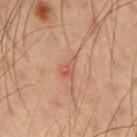{
  "biopsy_status": "not biopsied; imaged during a skin examination",
  "lesion_size": {
    "long_diameter_mm_approx": 2.5
  },
  "patient": {
    "sex": "male",
    "age_approx": 55
  },
  "site": "left upper arm",
  "image": {
    "source": "total-body photography crop",
    "field_of_view_mm": 15
  },
  "lighting": "cross-polarized"
}